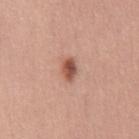Impression: No biopsy was performed on this lesion — it was imaged during a full skin examination and was not determined to be concerning. Context: A female subject about 50 years old. The lesion is located on the chest. Longest diameter approximately 3 mm. An algorithmic analysis of the crop reported a shape eccentricity near 0.8 and two-axis asymmetry of about 0.25. A lesion tile, about 15 mm wide, cut from a 3D total-body photograph. This is a white-light tile.